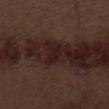The lesion was tiled from a total-body skin photograph and was not biopsied. Longest diameter approximately 2.5 mm. A 15 mm crop from a total-body photograph taken for skin-cancer surveillance. A male subject, roughly 70 years of age. From the abdomen. Imaged with white-light lighting.